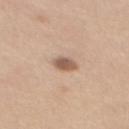follow-up — imaged on a skin check; not biopsied | TBP lesion metrics — a lesion color around L≈58 a*≈18 b*≈28 in CIELAB, about 13 CIELAB-L* units darker than the surrounding skin, and a lesion-to-skin contrast of about 8.5 (normalized; higher = more distinct); border irregularity of about 2 on a 0–10 scale, internal color variation of about 3 on a 0–10 scale, and peripheral color asymmetry of about 1; a classifier nevus-likeness of about 90/100 and a lesion-detection confidence of about 100/100 | subject — female, aged 38 to 42 | acquisition — ~15 mm crop, total-body skin-cancer survey | illumination — white-light illumination | anatomic site — the mid back | lesion size — ≈3 mm.